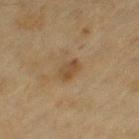Part of a total-body skin-imaging series; this lesion was reviewed on a skin check and was not flagged for biopsy. Longest diameter approximately 3 mm. From the left thigh. A lesion tile, about 15 mm wide, cut from a 3D total-body photograph. Automated image analysis of the tile measured a lesion area of about 4.5 mm², an outline eccentricity of about 0.85 (0 = round, 1 = elongated), and two-axis asymmetry of about 0.2. The analysis additionally found a border-irregularity rating of about 2/10, internal color variation of about 2 on a 0–10 scale, and a peripheral color-asymmetry measure near 1. It also reported a nevus-likeness score of about 10/100 and a detector confidence of about 100 out of 100 that the crop contains a lesion. The patient is a female in their mid- to late 50s.